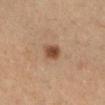Q: What is the imaging modality?
A: total-body-photography crop, ~15 mm field of view
Q: Patient demographics?
A: female, roughly 30 years of age
Q: Automated lesion metrics?
A: a footprint of about 5 mm², an outline eccentricity of about 0.65 (0 = round, 1 = elongated), and a shape-asymmetry score of about 0.15 (0 = symmetric); a lesion color around L≈43 a*≈18 b*≈29 in CIELAB, about 11 CIELAB-L* units darker than the surrounding skin, and a normalized border contrast of about 9; a border-irregularity index near 1.5/10 and peripheral color asymmetry of about 1; a classifier nevus-likeness of about 100/100
Q: Lesion size?
A: ≈2.5 mm
Q: Lesion location?
A: the leg
Q: What lighting was used for the tile?
A: cross-polarized illumination